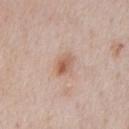{"biopsy_status": "not biopsied; imaged during a skin examination", "image": {"source": "total-body photography crop", "field_of_view_mm": 15}, "site": "chest", "lighting": "white-light", "patient": {"sex": "male", "age_approx": 60}}